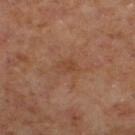Assessment: This lesion was catalogued during total-body skin photography and was not selected for biopsy. Clinical summary: The lesion is on the leg. The lesion-visualizer software estimated an area of roughly 4 mm² and a shape eccentricity near 0.65. The analysis additionally found an average lesion color of about L≈40 a*≈20 b*≈30 (CIELAB), roughly 5 lightness units darker than nearby skin, and a lesion-to-skin contrast of about 5 (normalized; higher = more distinct). It also reported a border-irregularity rating of about 2.5/10 and a within-lesion color-variation index near 2.5/10. The software also gave a classifier nevus-likeness of about 0/100 and a lesion-detection confidence of about 100/100. Imaged with cross-polarized lighting. The lesion's longest dimension is about 2.5 mm. A region of skin cropped from a whole-body photographic capture, roughly 15 mm wide. A male patient, approximately 60 years of age.